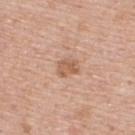Clinical impression: This lesion was catalogued during total-body skin photography and was not selected for biopsy. Background: Approximately 2.5 mm at its widest. Cropped from a whole-body photographic skin survey; the tile spans about 15 mm. The lesion is located on the upper back. Captured under white-light illumination. A male subject about 60 years old.